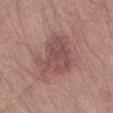Notes:
– biopsy status — no biopsy performed (imaged during a skin exam)
– tile lighting — white-light illumination
– patient — male, roughly 65 years of age
– size — about 6.5 mm
– image source — 15 mm crop, total-body photography
– automated metrics — an area of roughly 20 mm² and a symmetry-axis asymmetry near 0.4; an average lesion color of about L≈50 a*≈22 b*≈20 (CIELAB) and a lesion–skin lightness drop of about 9; a nevus-likeness score of about 0/100 and a lesion-detection confidence of about 100/100
– body site — the left thigh